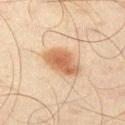{
  "biopsy_status": "not biopsied; imaged during a skin examination",
  "site": "left thigh",
  "automated_metrics": {
    "area_mm2_approx": 12.0,
    "eccentricity": 0.8,
    "shape_asymmetry": 0.15,
    "border_irregularity_0_10": 1.5,
    "peripheral_color_asymmetry": 1.0,
    "nevus_likeness_0_100": 100,
    "lesion_detection_confidence_0_100": 100
  },
  "patient": {
    "sex": "male",
    "age_approx": 45
  },
  "lighting": "cross-polarized",
  "image": {
    "source": "total-body photography crop",
    "field_of_view_mm": 15
  },
  "lesion_size": {
    "long_diameter_mm_approx": 5.0
  }
}